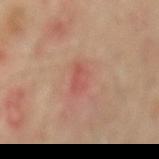- workup · catalogued during a skin exam; not biopsied
- patient · male, in their mid- to late 60s
- lesion size · ~3 mm (longest diameter)
- acquisition · 15 mm crop, total-body photography
- site · the lower back